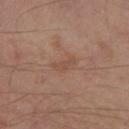<lesion>
  <biopsy_status>not biopsied; imaged during a skin examination</biopsy_status>
  <image>
    <source>total-body photography crop</source>
    <field_of_view_mm>15</field_of_view_mm>
  </image>
  <patient>
    <age_approx>55</age_approx>
  </patient>
  <site>left lower leg</site>
</lesion>